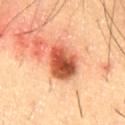<case>
  <lighting>cross-polarized</lighting>
  <image>
    <source>total-body photography crop</source>
    <field_of_view_mm>15</field_of_view_mm>
  </image>
  <patient>
    <sex>male</sex>
    <age_approx>55</age_approx>
  </patient>
  <lesion_size>
    <long_diameter_mm_approx>4.5</long_diameter_mm_approx>
  </lesion_size>
  <site>upper back</site>
  <automated_metrics>
    <cielab_L>49</cielab_L>
    <cielab_a>28</cielab_a>
    <cielab_b>34</cielab_b>
    <vs_skin_darker_L>17.0</vs_skin_darker_L>
  </automated_metrics>
</case>